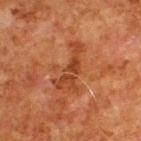{
  "biopsy_status": "not biopsied; imaged during a skin examination",
  "image": {
    "source": "total-body photography crop",
    "field_of_view_mm": 15
  },
  "patient": {
    "sex": "male",
    "age_approx": 80
  },
  "automated_metrics": {
    "area_mm2_approx": 10.0,
    "eccentricity": 0.9,
    "shape_asymmetry": 0.6,
    "vs_skin_darker_L": 8.0,
    "border_irregularity_0_10": 7.5,
    "color_variation_0_10": 3.0,
    "peripheral_color_asymmetry": 1.0
  },
  "lighting": "cross-polarized",
  "site": "upper back"
}